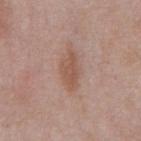Notes:
– follow-up — no biopsy performed (imaged during a skin exam)
– diameter — about 4 mm
– lighting — white-light illumination
– location — the chest
– patient — male, approximately 55 years of age
– image source — ~15 mm tile from a whole-body skin photo